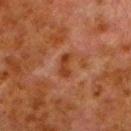Imaged during a routine full-body skin examination; the lesion was not biopsied and no histopathology is available. On the left lower leg. This is a cross-polarized tile. Measured at roughly 3 mm in maximum diameter. Automated tile analysis of the lesion measured an eccentricity of roughly 0.9 and a shape-asymmetry score of about 0.3 (0 = symmetric). The software also gave a mean CIELAB color near L≈31 a*≈23 b*≈30, a lesion–skin lightness drop of about 7, and a normalized border contrast of about 7.5. The software also gave a border-irregularity index near 3.5/10 and a color-variation rating of about 2.5/10. A male patient aged 78–82. A 15 mm close-up extracted from a 3D total-body photography capture.